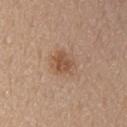| field | value |
|---|---|
| follow-up | total-body-photography surveillance lesion; no biopsy |
| body site | the right upper arm |
| lighting | white-light illumination |
| subject | female, aged around 65 |
| size | ~3 mm (longest diameter) |
| TBP lesion metrics | an eccentricity of roughly 0.55 and a shape-asymmetry score of about 0.2 (0 = symmetric); a border-irregularity index near 1.5/10, internal color variation of about 3 on a 0–10 scale, and peripheral color asymmetry of about 1 |
| imaging modality | total-body-photography crop, ~15 mm field of view |